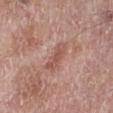Part of a total-body skin-imaging series; this lesion was reviewed on a skin check and was not flagged for biopsy. The lesion is located on the right lower leg. A male patient, about 75 years old. The lesion-visualizer software estimated a lesion color around L≈53 a*≈23 b*≈26 in CIELAB and a lesion-to-skin contrast of about 6 (normalized; higher = more distinct). Imaged with white-light lighting. Cropped from a total-body skin-imaging series; the visible field is about 15 mm. Measured at roughly 4 mm in maximum diameter.